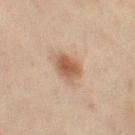| key | value |
|---|---|
| workup | imaged on a skin check; not biopsied |
| automated lesion analysis | an automated nevus-likeness rating near 100 out of 100 and lesion-presence confidence of about 100/100 |
| lesion diameter | about 3.5 mm |
| illumination | cross-polarized illumination |
| imaging modality | ~15 mm crop, total-body skin-cancer survey |
| patient | female, in their mid- to late 40s |
| site | the left thigh |